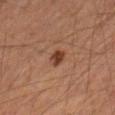Clinical impression:
Recorded during total-body skin imaging; not selected for excision or biopsy.
Image and clinical context:
The tile uses cross-polarized illumination. On the right thigh. The patient is a male aged approximately 65. The lesion's longest dimension is about 2 mm. Cropped from a whole-body photographic skin survey; the tile spans about 15 mm.